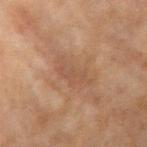Impression:
Recorded during total-body skin imaging; not selected for excision or biopsy.
Acquisition and patient details:
A roughly 15 mm field-of-view crop from a total-body skin photograph. The lesion is on the arm. The lesion's longest dimension is about 5 mm. Captured under cross-polarized illumination. A female subject approximately 55 years of age.